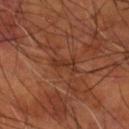follow-up: total-body-photography surveillance lesion; no biopsy
tile lighting: cross-polarized illumination
site: the right forearm
size: ≈3 mm
image: ~15 mm tile from a whole-body skin photo
patient: male, in their mid- to late 50s